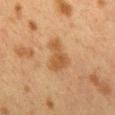Clinical impression: Recorded during total-body skin imaging; not selected for excision or biopsy. Background: Imaged with cross-polarized lighting. A female patient, approximately 40 years of age. A roughly 15 mm field-of-view crop from a total-body skin photograph. The total-body-photography lesion software estimated a footprint of about 8.5 mm² and an eccentricity of roughly 0.8. And it measured a border-irregularity rating of about 4.5/10. About 4 mm across. The lesion is located on the mid back.